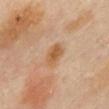Findings:
• notes: imaged on a skin check; not biopsied
• subject: female, aged 58 to 62
• image: total-body-photography crop, ~15 mm field of view
• diameter: ~3 mm (longest diameter)
• image-analysis metrics: an area of roughly 6 mm² and two-axis asymmetry of about 0.15; a lesion color around L≈59 a*≈21 b*≈39 in CIELAB and about 9 CIELAB-L* units darker than the surrounding skin; internal color variation of about 3.5 on a 0–10 scale and a peripheral color-asymmetry measure near 1; a nevus-likeness score of about 35/100
• site: the mid back